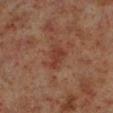The patient is a male aged 58–62.
The lesion is on the left lower leg.
This is a cross-polarized tile.
About 3 mm across.
A region of skin cropped from a whole-body photographic capture, roughly 15 mm wide.